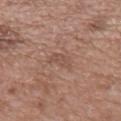Captured during whole-body skin photography for melanoma surveillance; the lesion was not biopsied.
A close-up tile cropped from a whole-body skin photograph, about 15 mm across.
On the mid back.
The patient is a male aged approximately 50.
Longest diameter approximately 3 mm.
An algorithmic analysis of the crop reported an area of roughly 4.5 mm² and a symmetry-axis asymmetry near 0.4. The software also gave a border-irregularity index near 4/10, a color-variation rating of about 1/10, and radial color variation of about 0.5. The software also gave a classifier nevus-likeness of about 0/100 and a lesion-detection confidence of about 100/100.